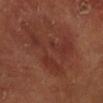notes: imaged on a skin check; not biopsied
patient: male, aged approximately 55
body site: the left lower leg
image source: ~15 mm crop, total-body skin-cancer survey